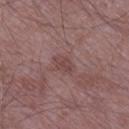workup: no biopsy performed (imaged during a skin exam) | image: total-body-photography crop, ~15 mm field of view | body site: the right lower leg | patient: male, in their 50s.